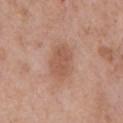lesion size: about 4 mm; body site: the chest; acquisition: ~15 mm crop, total-body skin-cancer survey; patient: male, aged approximately 70.